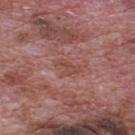The lesion was photographed on a routine skin check and not biopsied; there is no pathology result. A male subject, in their 70s. A lesion tile, about 15 mm wide, cut from a 3D total-body photograph. Approximately 3.5 mm at its widest. Automated image analysis of the tile measured a footprint of about 4.5 mm², an outline eccentricity of about 0.85 (0 = round, 1 = elongated), and two-axis asymmetry of about 0.4. The analysis additionally found a mean CIELAB color near L≈47 a*≈25 b*≈24, about 6 CIELAB-L* units darker than the surrounding skin, and a normalized border contrast of about 4.5. The software also gave border irregularity of about 5 on a 0–10 scale, a within-lesion color-variation index near 1/10, and radial color variation of about 0. Located on the back.